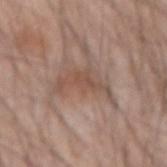Background:
The lesion is located on the right forearm. Measured at roughly 5.5 mm in maximum diameter. Cropped from a whole-body photographic skin survey; the tile spans about 15 mm. This is a white-light tile. The patient is a male in their mid- to late 40s.